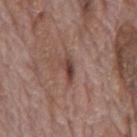Assessment: Recorded during total-body skin imaging; not selected for excision or biopsy. Acquisition and patient details: About 3 mm across. The lesion is on the back. A male subject in their 70s. This image is a 15 mm lesion crop taken from a total-body photograph. Captured under white-light illumination.